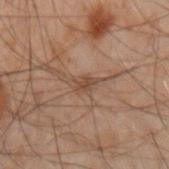<case>
  <biopsy_status>not biopsied; imaged during a skin examination</biopsy_status>
  <patient>
    <sex>male</sex>
    <age_approx>50</age_approx>
  </patient>
  <image>
    <source>total-body photography crop</source>
    <field_of_view_mm>15</field_of_view_mm>
  </image>
  <site>arm</site>
</case>